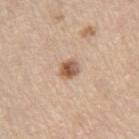The lesion was photographed on a routine skin check and not biopsied; there is no pathology result. A male subject, roughly 70 years of age. A region of skin cropped from a whole-body photographic capture, roughly 15 mm wide. On the right thigh. The lesion-visualizer software estimated a lesion color around L≈57 a*≈18 b*≈31 in CIELAB, a lesion–skin lightness drop of about 14, and a normalized lesion–skin contrast near 9.5. It also reported an automated nevus-likeness rating near 90 out of 100. Longest diameter approximately 2.5 mm. Captured under white-light illumination.